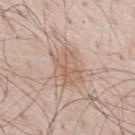This lesion was catalogued during total-body skin photography and was not selected for biopsy.
Imaged with white-light lighting.
About 4.5 mm across.
A male patient aged approximately 80.
A close-up tile cropped from a whole-body skin photograph, about 15 mm across.
The lesion is on the mid back.
An algorithmic analysis of the crop reported a border-irregularity rating of about 3.5/10, internal color variation of about 4 on a 0–10 scale, and peripheral color asymmetry of about 1.5.